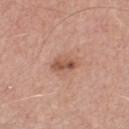Clinical impression: Imaged during a routine full-body skin examination; the lesion was not biopsied and no histopathology is available. Clinical summary: The tile uses white-light illumination. From the front of the torso. The patient is a male approximately 60 years of age. Cropped from a whole-body photographic skin survey; the tile spans about 15 mm. Longest diameter approximately 3.5 mm.